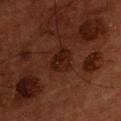The lesion was tiled from a total-body skin photograph and was not biopsied. A region of skin cropped from a whole-body photographic capture, roughly 15 mm wide. The total-body-photography lesion software estimated a lesion-to-skin contrast of about 8 (normalized; higher = more distinct). And it measured a nevus-likeness score of about 90/100 and a lesion-detection confidence of about 100/100. The lesion's longest dimension is about 3 mm. This is a cross-polarized tile. The lesion is located on the chest. A male subject aged 53–57.